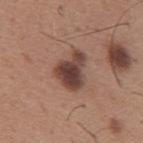| field | value |
|---|---|
| anatomic site | the mid back |
| lighting | white-light illumination |
| patient | male, aged approximately 65 |
| image source | ~15 mm crop, total-body skin-cancer survey |
| size | ~4.5 mm (longest diameter) |